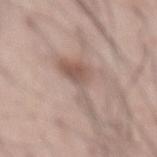Part of a total-body skin-imaging series; this lesion was reviewed on a skin check and was not flagged for biopsy. On the abdomen. Imaged with white-light lighting. Automated tile analysis of the lesion measured border irregularity of about 9.5 on a 0–10 scale, a color-variation rating of about 3/10, and radial color variation of about 1. The software also gave a nevus-likeness score of about 0/100 and a detector confidence of about 70 out of 100 that the crop contains a lesion. A male subject aged approximately 55. Measured at roughly 7 mm in maximum diameter. A region of skin cropped from a whole-body photographic capture, roughly 15 mm wide.